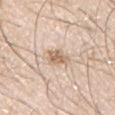Case summary:
• workup — catalogued during a skin exam; not biopsied
• patient — male, approximately 30 years of age
• image source — ~15 mm crop, total-body skin-cancer survey
• location — the left upper arm